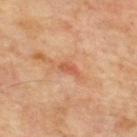Assessment:
No biopsy was performed on this lesion — it was imaged during a full skin examination and was not determined to be concerning.
Image and clinical context:
Located on the upper back. A 15 mm crop from a total-body photograph taken for skin-cancer surveillance. The patient is a male approximately 55 years of age. Captured under cross-polarized illumination. Longest diameter approximately 2.5 mm.